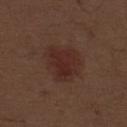Impression: This lesion was catalogued during total-body skin photography and was not selected for biopsy. Context: This is a white-light tile. A male subject about 70 years old. The lesion is on the abdomen. About 5 mm across. Automated tile analysis of the lesion measured a footprint of about 14 mm², a shape eccentricity near 0.55, and two-axis asymmetry of about 0.3. The software also gave an average lesion color of about L≈27 a*≈20 b*≈22 (CIELAB) and about 6 CIELAB-L* units darker than the surrounding skin. The analysis additionally found a border-irregularity rating of about 3/10, a within-lesion color-variation index near 2.5/10, and a peripheral color-asymmetry measure near 1. The analysis additionally found an automated nevus-likeness rating near 45 out of 100. A 15 mm close-up tile from a total-body photography series done for melanoma screening.